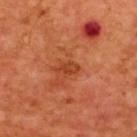diameter = ~3 mm (longest diameter); image source = total-body-photography crop, ~15 mm field of view; subject = male, aged approximately 65; location = the upper back.